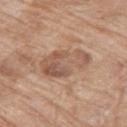Recorded during total-body skin imaging; not selected for excision or biopsy.
A roughly 15 mm field-of-view crop from a total-body skin photograph.
Imaged with white-light lighting.
A female patient in their mid- to late 70s.
On the right thigh.
An algorithmic analysis of the crop reported a border-irregularity rating of about 4/10 and peripheral color asymmetry of about 2. The software also gave a lesion-detection confidence of about 100/100.